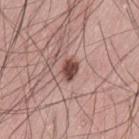Findings:
- follow-up: total-body-photography surveillance lesion; no biopsy
- automated lesion analysis: a mean CIELAB color near L≈47 a*≈22 b*≈23, roughly 15 lightness units darker than nearby skin, and a lesion-to-skin contrast of about 10.5 (normalized; higher = more distinct); a border-irregularity index near 2/10, a within-lesion color-variation index near 2.5/10, and a peripheral color-asymmetry measure near 1; an automated nevus-likeness rating near 95 out of 100 and lesion-presence confidence of about 100/100
- image source: 15 mm crop, total-body photography
- patient: male, about 60 years old
- tile lighting: white-light
- body site: the left thigh